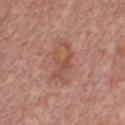Captured during whole-body skin photography for melanoma surveillance; the lesion was not biopsied.
Located on the chest.
The patient is a male aged around 70.
A 15 mm crop from a total-body photograph taken for skin-cancer surveillance.